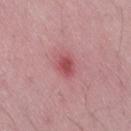{"biopsy_status": "not biopsied; imaged during a skin examination"}